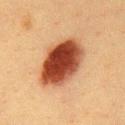Clinical impression: Captured during whole-body skin photography for melanoma surveillance; the lesion was not biopsied. Image and clinical context: Cropped from a whole-body photographic skin survey; the tile spans about 15 mm. A female patient, roughly 40 years of age. The lesion is located on the chest. This is a cross-polarized tile. The recorded lesion diameter is about 7 mm.